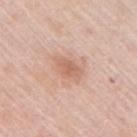Clinical impression:
Part of a total-body skin-imaging series; this lesion was reviewed on a skin check and was not flagged for biopsy.
Clinical summary:
A 15 mm crop from a total-body photograph taken for skin-cancer surveillance. Imaged with white-light lighting. Longest diameter approximately 3.5 mm. From the right upper arm. A female subject aged 63 to 67.